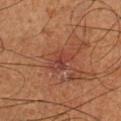No biopsy was performed on this lesion — it was imaged during a full skin examination and was not determined to be concerning.
From the left lower leg.
The lesion-visualizer software estimated an outline eccentricity of about 0.75 (0 = round, 1 = elongated).
A male subject aged 63–67.
Captured under cross-polarized illumination.
Cropped from a whole-body photographic skin survey; the tile spans about 15 mm.